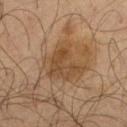Clinical impression: The lesion was photographed on a routine skin check and not biopsied; there is no pathology result. Background: On the right thigh. The lesion-visualizer software estimated a footprint of about 15 mm², a shape eccentricity near 0.65, and a symmetry-axis asymmetry near 0.45. The software also gave border irregularity of about 5 on a 0–10 scale. It also reported a nevus-likeness score of about 5/100 and a detector confidence of about 100 out of 100 that the crop contains a lesion. This image is a 15 mm lesion crop taken from a total-body photograph. A male subject aged 58 to 62. The lesion's longest dimension is about 5 mm.